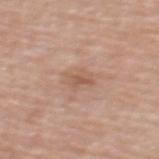workup: no biopsy performed (imaged during a skin exam) | illumination: white-light illumination | patient: female, in their mid- to late 50s | image: total-body-photography crop, ~15 mm field of view | body site: the upper back | diameter: ≈3 mm.